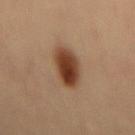Assessment:
This lesion was catalogued during total-body skin photography and was not selected for biopsy.
Acquisition and patient details:
The recorded lesion diameter is about 5 mm. The patient is a male aged 38 to 42. On the mid back. A 15 mm crop from a total-body photograph taken for skin-cancer surveillance. This is a cross-polarized tile.